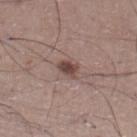– lighting: white-light
– subject: male, aged 48 to 52
– anatomic site: the right lower leg
– diameter: ≈2.5 mm
– acquisition: total-body-photography crop, ~15 mm field of view